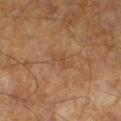  image:
    source: total-body photography crop
    field_of_view_mm: 15
  patient:
    sex: male
    age_approx: 65
  lighting: cross-polarized